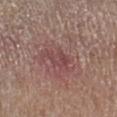Clinical impression: Imaged during a routine full-body skin examination; the lesion was not biopsied and no histopathology is available. Clinical summary: A male subject, approximately 65 years of age. An algorithmic analysis of the crop reported an average lesion color of about L≈45 a*≈23 b*≈19 (CIELAB), roughly 7 lightness units darker than nearby skin, and a normalized lesion–skin contrast near 5.5. It also reported a border-irregularity index near 4/10, internal color variation of about 2.5 on a 0–10 scale, and peripheral color asymmetry of about 1. And it measured a detector confidence of about 100 out of 100 that the crop contains a lesion. Located on the left lower leg. The lesion's longest dimension is about 3 mm. This is a white-light tile. A close-up tile cropped from a whole-body skin photograph, about 15 mm across.